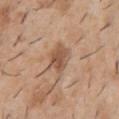Part of a total-body skin-imaging series; this lesion was reviewed on a skin check and was not flagged for biopsy. The total-body-photography lesion software estimated an area of roughly 9.5 mm², an outline eccentricity of about 0.75 (0 = round, 1 = elongated), and a shape-asymmetry score of about 0.35 (0 = symmetric). The software also gave a border-irregularity index near 4/10 and peripheral color asymmetry of about 1.5. The software also gave a nevus-likeness score of about 10/100. A male patient, aged 38–42. Imaged with white-light lighting. Longest diameter approximately 4.5 mm. A 15 mm crop from a total-body photograph taken for skin-cancer surveillance. The lesion is located on the chest.